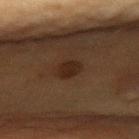site = the chest
imaging modality = 15 mm crop, total-body photography
patient = male, aged around 85 or older